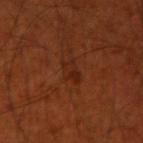biopsy status = total-body-photography surveillance lesion; no biopsy
location = the right upper arm
tile lighting = cross-polarized illumination
image = ~15 mm tile from a whole-body skin photo
patient = male, aged 68 to 72